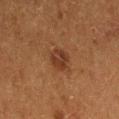| feature | finding |
|---|---|
| subject | female, in their 50s |
| acquisition | total-body-photography crop, ~15 mm field of view |
| TBP lesion metrics | a lesion area of about 6.5 mm², an outline eccentricity of about 0.4 (0 = round, 1 = elongated), and a symmetry-axis asymmetry near 0.15; a mean CIELAB color near L≈30 a*≈19 b*≈27, a lesion–skin lightness drop of about 7, and a lesion-to-skin contrast of about 7 (normalized; higher = more distinct); a border-irregularity index near 1.5/10, a within-lesion color-variation index near 2.5/10, and a peripheral color-asymmetry measure near 1; an automated nevus-likeness rating near 60 out of 100 and lesion-presence confidence of about 100/100 |
| diameter | ~3 mm (longest diameter) |
| illumination | cross-polarized |
| body site | the lower back |